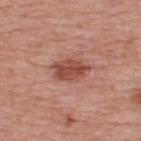The lesion was photographed on a routine skin check and not biopsied; there is no pathology result. The lesion is located on the back. Longest diameter approximately 4 mm. A male patient aged around 75. A roughly 15 mm field-of-view crop from a total-body skin photograph. Captured under white-light illumination.